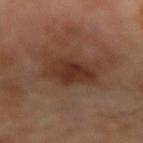This lesion was catalogued during total-body skin photography and was not selected for biopsy. Located on the left forearm. The subject is a male aged 68–72. Automated tile analysis of the lesion measured a footprint of about 14 mm² and two-axis asymmetry of about 0.2. The analysis additionally found a lesion color around L≈32 a*≈19 b*≈26 in CIELAB, roughly 9 lightness units darker than nearby skin, and a lesion-to-skin contrast of about 8.5 (normalized; higher = more distinct). This image is a 15 mm lesion crop taken from a total-body photograph. About 5.5 mm across. The tile uses cross-polarized illumination.